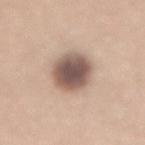On the lower back. A region of skin cropped from a whole-body photographic capture, roughly 15 mm wide. The subject is a male about 50 years old. This is a white-light tile. Measured at roughly 5.5 mm in maximum diameter.